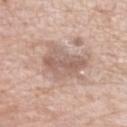This lesion was catalogued during total-body skin photography and was not selected for biopsy. The lesion is located on the left forearm. A female subject aged 63–67. A roughly 15 mm field-of-view crop from a total-body skin photograph. The lesion-visualizer software estimated an area of roughly 14 mm², a shape eccentricity near 0.4, and two-axis asymmetry of about 0.3. And it measured an average lesion color of about L≈60 a*≈17 b*≈24 (CIELAB), a lesion–skin lightness drop of about 9, and a lesion-to-skin contrast of about 6 (normalized; higher = more distinct). It also reported border irregularity of about 3.5 on a 0–10 scale. And it measured a detector confidence of about 100 out of 100 that the crop contains a lesion. This is a white-light tile. The recorded lesion diameter is about 4.5 mm.